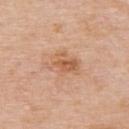* biopsy status — total-body-photography surveillance lesion; no biopsy
* imaging modality — 15 mm crop, total-body photography
* lesion diameter — ~4.5 mm (longest diameter)
* site — the upper back
* image-analysis metrics — an area of roughly 9 mm², a shape eccentricity near 0.7, and a symmetry-axis asymmetry near 0.25; a lesion color around L≈61 a*≈22 b*≈34 in CIELAB, roughly 9 lightness units darker than nearby skin, and a normalized lesion–skin contrast near 6; a border-irregularity index near 3.5/10, a color-variation rating of about 5/10, and peripheral color asymmetry of about 1.5
* subject — male, roughly 75 years of age
* tile lighting — white-light illumination